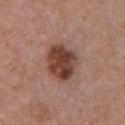Part of a total-body skin-imaging series; this lesion was reviewed on a skin check and was not flagged for biopsy. The lesion's longest dimension is about 4.5 mm. This image is a 15 mm lesion crop taken from a total-body photograph. The tile uses white-light illumination. On the chest. A female patient, in their mid-30s.